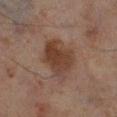The lesion was tiled from a total-body skin photograph and was not biopsied. The lesion is on the left lower leg. The subject is a male in their 70s. Imaged with cross-polarized lighting. A 15 mm crop from a total-body photograph taken for skin-cancer surveillance.